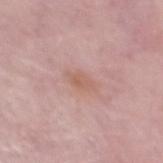workup = imaged on a skin check; not biopsied | image = ~15 mm tile from a whole-body skin photo | size = about 3 mm | site = the front of the torso | patient = female, aged 58 to 62 | lighting = white-light illumination | automated lesion analysis = a footprint of about 3.5 mm², an eccentricity of roughly 0.85, and a symmetry-axis asymmetry near 0.25; a border-irregularity index near 2.5/10, internal color variation of about 2 on a 0–10 scale, and peripheral color asymmetry of about 1.